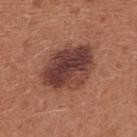biopsy status=total-body-photography surveillance lesion; no biopsy | body site=the chest | patient=female, roughly 35 years of age | diameter=≈6.5 mm | lighting=white-light illumination | image source=15 mm crop, total-body photography.